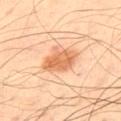notes = catalogued during a skin exam; not biopsied
tile lighting = cross-polarized
subject = male, aged around 50
site = the upper back
diameter = ~4 mm (longest diameter)
image = 15 mm crop, total-body photography
automated metrics = a footprint of about 10 mm², an eccentricity of roughly 0.65, and two-axis asymmetry of about 0.2; a lesion–skin lightness drop of about 12 and a normalized border contrast of about 7.5; a border-irregularity index near 2.5/10; a classifier nevus-likeness of about 95/100